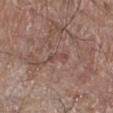Case summary:
• notes · total-body-photography surveillance lesion; no biopsy
• imaging modality · ~15 mm tile from a whole-body skin photo
• lighting · white-light illumination
• lesion size · about 3 mm
• subject · male, in their mid- to late 60s
• site · the right lower leg
• automated metrics · a mean CIELAB color near L≈46 a*≈19 b*≈23, a lesion–skin lightness drop of about 7, and a lesion-to-skin contrast of about 5.5 (normalized; higher = more distinct); border irregularity of about 6 on a 0–10 scale, a within-lesion color-variation index near 0/10, and radial color variation of about 0; an automated nevus-likeness rating near 0 out of 100 and a detector confidence of about 55 out of 100 that the crop contains a lesion